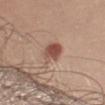The lesion was tiled from a total-body skin photograph and was not biopsied. On the left upper arm. A region of skin cropped from a whole-body photographic capture, roughly 15 mm wide. A male patient in their mid- to late 50s.